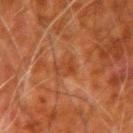No biopsy was performed on this lesion — it was imaged during a full skin examination and was not determined to be concerning. A male patient in their 80s. The lesion is located on the left upper arm. Cropped from a whole-body photographic skin survey; the tile spans about 15 mm.